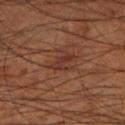biopsy status=total-body-photography surveillance lesion; no biopsy
acquisition=~15 mm crop, total-body skin-cancer survey
subject=male, aged 53–57
body site=the leg